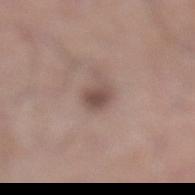Assessment:
Imaged during a routine full-body skin examination; the lesion was not biopsied and no histopathology is available.
Context:
This is a white-light tile. A lesion tile, about 15 mm wide, cut from a 3D total-body photograph. The recorded lesion diameter is about 2.5 mm. The subject is a male in their 60s. Automated tile analysis of the lesion measured a mean CIELAB color near L≈49 a*≈16 b*≈22, roughly 11 lightness units darker than nearby skin, and a normalized lesion–skin contrast near 8. The lesion is located on the left lower leg.